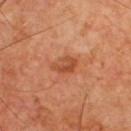Image and clinical context: Captured under cross-polarized illumination. The lesion's longest dimension is about 3 mm. The lesion is on the chest. A male subject aged approximately 65. Cropped from a total-body skin-imaging series; the visible field is about 15 mm.